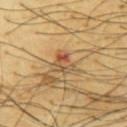No biopsy was performed on this lesion — it was imaged during a full skin examination and was not determined to be concerning.